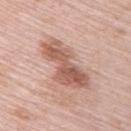biopsy_status: not biopsied; imaged during a skin examination
lighting: white-light
site: upper back
automated_metrics:
  area_mm2_approx: 18.0
  cielab_L: 58
  cielab_a: 22
  cielab_b: 28
  vs_skin_darker_L: 13.0
  border_irregularity_0_10: 5.5
  color_variation_0_10: 5.5
  peripheral_color_asymmetry: 2.0
  nevus_likeness_0_100: 0
  lesion_detection_confidence_0_100: 100
image:
  source: total-body photography crop
  field_of_view_mm: 15
lesion_size:
  long_diameter_mm_approx: 7.5
patient:
  sex: female
  age_approx: 65